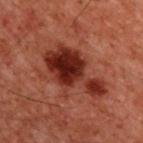  biopsy_status: not biopsied; imaged during a skin examination
  patient:
    sex: male
    age_approx: 60
  lighting: cross-polarized
  image:
    source: total-body photography crop
    field_of_view_mm: 15
  automated_metrics:
    eccentricity: 0.8
    shape_asymmetry: 0.4
    vs_skin_darker_L: 11.0
    vs_skin_contrast_norm: 11.0
  lesion_size:
    long_diameter_mm_approx: 7.5
  site: upper back